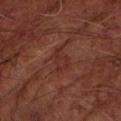{
  "biopsy_status": "not biopsied; imaged during a skin examination",
  "lighting": "cross-polarized",
  "site": "left forearm",
  "image": {
    "source": "total-body photography crop",
    "field_of_view_mm": 15
  },
  "patient": {
    "sex": "male",
    "age_approx": 65
  },
  "lesion_size": {
    "long_diameter_mm_approx": 3.0
  },
  "automated_metrics": {
    "eccentricity": 0.75,
    "shape_asymmetry": 0.35,
    "cielab_L": 28,
    "cielab_a": 24,
    "cielab_b": 24,
    "vs_skin_darker_L": 5.0,
    "vs_skin_contrast_norm": 5.0,
    "color_variation_0_10": 1.5,
    "peripheral_color_asymmetry": 0.5
  }
}